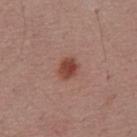Imaged during a routine full-body skin examination; the lesion was not biopsied and no histopathology is available. From the mid back. A male subject aged 53 to 57. This is a white-light tile. The recorded lesion diameter is about 3 mm. A 15 mm close-up extracted from a 3D total-body photography capture.